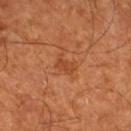No biopsy was performed on this lesion — it was imaged during a full skin examination and was not determined to be concerning.